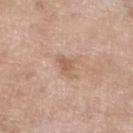The lesion was tiled from a total-body skin photograph and was not biopsied.
Located on the left lower leg.
This is a white-light tile.
A female subject roughly 75 years of age.
Approximately 3 mm at its widest.
A region of skin cropped from a whole-body photographic capture, roughly 15 mm wide.